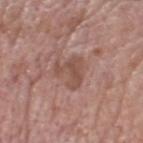  biopsy_status: not biopsied; imaged during a skin examination
  image:
    source: total-body photography crop
    field_of_view_mm: 15
  site: head or neck
  lesion_size:
    long_diameter_mm_approx: 4.0
  patient:
    sex: male
    age_approx: 70